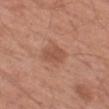{"biopsy_status": "not biopsied; imaged during a skin examination", "patient": {"sex": "male", "age_approx": 65}, "site": "left thigh", "image": {"source": "total-body photography crop", "field_of_view_mm": 15}}